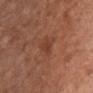• follow-up: imaged on a skin check; not biopsied
• tile lighting: white-light
• image: ~15 mm crop, total-body skin-cancer survey
• subject: female, in their 40s
• site: the chest
• lesion diameter: about 3 mm
• automated lesion analysis: a lesion area of about 4 mm² and a symmetry-axis asymmetry near 0.3